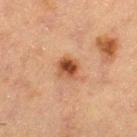Impression: Captured during whole-body skin photography for melanoma surveillance; the lesion was not biopsied. Acquisition and patient details: The recorded lesion diameter is about 3 mm. A male subject, aged approximately 65. This is a cross-polarized tile. The lesion is located on the right thigh. A 15 mm close-up extracted from a 3D total-body photography capture.